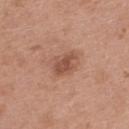Q: Is there a histopathology result?
A: catalogued during a skin exam; not biopsied
Q: Who is the patient?
A: female, about 40 years old
Q: How was this image acquired?
A: total-body-photography crop, ~15 mm field of view
Q: Where on the body is the lesion?
A: the upper back